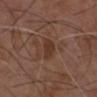This lesion was catalogued during total-body skin photography and was not selected for biopsy. Captured under white-light illumination. The lesion's longest dimension is about 3.5 mm. A male patient, aged approximately 75. From the chest. A 15 mm crop from a total-body photograph taken for skin-cancer surveillance. Automated image analysis of the tile measured a lesion color around L≈35 a*≈19 b*≈26 in CIELAB.